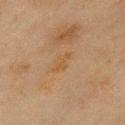Part of a total-body skin-imaging series; this lesion was reviewed on a skin check and was not flagged for biopsy.
Measured at roughly 3 mm in maximum diameter.
A 15 mm crop from a total-body photograph taken for skin-cancer surveillance.
A male subject in their mid- to late 40s.
Automated image analysis of the tile measured an area of roughly 3.5 mm², an outline eccentricity of about 0.85 (0 = round, 1 = elongated), and a symmetry-axis asymmetry near 0.4. It also reported an average lesion color of about L≈43 a*≈15 b*≈32 (CIELAB), a lesion–skin lightness drop of about 4, and a normalized border contrast of about 5.5. It also reported a classifier nevus-likeness of about 0/100 and a lesion-detection confidence of about 100/100.
Imaged with cross-polarized lighting.
On the chest.